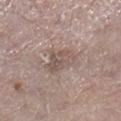follow-up: no biopsy performed (imaged during a skin exam)
image-analysis metrics: a shape eccentricity near 0.85 and a symmetry-axis asymmetry near 0.35; an average lesion color of about L≈53 a*≈14 b*≈21 (CIELAB), roughly 8 lightness units darker than nearby skin, and a lesion-to-skin contrast of about 6 (normalized; higher = more distinct)
patient: male, in their mid- to late 60s
location: the right lower leg
lighting: white-light
lesion diameter: ~4 mm (longest diameter)
image source: ~15 mm crop, total-body skin-cancer survey